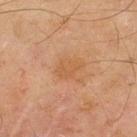Assessment: This lesion was catalogued during total-body skin photography and was not selected for biopsy. Background: From the upper back. The total-body-photography lesion software estimated an average lesion color of about L≈45 a*≈18 b*≈32 (CIELAB), about 5 CIELAB-L* units darker than the surrounding skin, and a lesion-to-skin contrast of about 5 (normalized; higher = more distinct). The software also gave border irregularity of about 2.5 on a 0–10 scale, a color-variation rating of about 1/10, and peripheral color asymmetry of about 0.5. Cropped from a total-body skin-imaging series; the visible field is about 15 mm. A male subject, roughly 45 years of age. The lesion's longest dimension is about 3.5 mm.